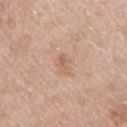biopsy status = catalogued during a skin exam; not biopsied
body site = the upper back
illumination = white-light
image = total-body-photography crop, ~15 mm field of view
TBP lesion metrics = a mean CIELAB color near L≈61 a*≈21 b*≈30 and roughly 7 lightness units darker than nearby skin; a border-irregularity rating of about 4/10 and a color-variation rating of about 0.5/10
subject = female, aged approximately 45
size = about 2.5 mm